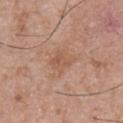{"biopsy_status": "not biopsied; imaged during a skin examination", "image": {"source": "total-body photography crop", "field_of_view_mm": 15}, "site": "chest", "patient": {"sex": "male", "age_approx": 50}}